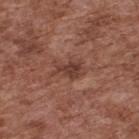Imaged during a routine full-body skin examination; the lesion was not biopsied and no histopathology is available. A lesion tile, about 15 mm wide, cut from a 3D total-body photograph. Measured at roughly 3.5 mm in maximum diameter. A male subject, approximately 75 years of age. Located on the upper back.